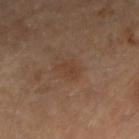No biopsy was performed on this lesion — it was imaged during a full skin examination and was not determined to be concerning. An algorithmic analysis of the crop reported an area of roughly 4 mm², a shape eccentricity near 0.75, and a shape-asymmetry score of about 0.35 (0 = symmetric). The software also gave a border-irregularity rating of about 4/10 and internal color variation of about 1 on a 0–10 scale. It also reported a nevus-likeness score of about 0/100. A 15 mm crop from a total-body photograph taken for skin-cancer surveillance. The lesion is located on the arm. A female subject aged approximately 60. Captured under cross-polarized illumination.